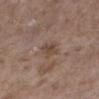Q: Is there a histopathology result?
A: catalogued during a skin exam; not biopsied
Q: What kind of image is this?
A: total-body-photography crop, ~15 mm field of view
Q: Automated lesion metrics?
A: a lesion area of about 4.5 mm², an outline eccentricity of about 0.75 (0 = round, 1 = elongated), and two-axis asymmetry of about 0.35; border irregularity of about 3.5 on a 0–10 scale and a peripheral color-asymmetry measure near 1; an automated nevus-likeness rating near 0 out of 100
Q: Patient demographics?
A: female, aged approximately 65
Q: Where on the body is the lesion?
A: the left lower leg